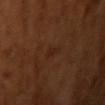Impression:
The lesion was tiled from a total-body skin photograph and was not biopsied.
Background:
The recorded lesion diameter is about 2.5 mm. Automated tile analysis of the lesion measured an area of roughly 2 mm², a shape eccentricity near 0.95, and a symmetry-axis asymmetry near 0.5. The analysis additionally found a lesion color around L≈24 a*≈20 b*≈27 in CIELAB, a lesion–skin lightness drop of about 4, and a normalized lesion–skin contrast near 5. The software also gave internal color variation of about 0 on a 0–10 scale. The lesion is located on the right upper arm. A lesion tile, about 15 mm wide, cut from a 3D total-body photograph. A female patient, roughly 55 years of age.